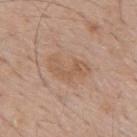{"biopsy_status": "not biopsied; imaged during a skin examination", "site": "mid back", "image": {"source": "total-body photography crop", "field_of_view_mm": 15}, "patient": {"sex": "male", "age_approx": 60}}